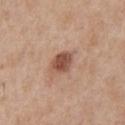Q: Is there a histopathology result?
A: catalogued during a skin exam; not biopsied
Q: Patient demographics?
A: male, about 65 years old
Q: Automated lesion metrics?
A: an average lesion color of about L≈50 a*≈23 b*≈29 (CIELAB) and a lesion-to-skin contrast of about 9.5 (normalized; higher = more distinct); border irregularity of about 2 on a 0–10 scale, a within-lesion color-variation index near 3.5/10, and radial color variation of about 1; a classifier nevus-likeness of about 90/100
Q: What kind of image is this?
A: total-body-photography crop, ~15 mm field of view
Q: What is the anatomic site?
A: the front of the torso